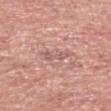{"biopsy_status": "not biopsied; imaged during a skin examination", "lesion_size": {"long_diameter_mm_approx": 3.5}, "lighting": "white-light", "patient": {"sex": "male", "age_approx": 65}, "image": {"source": "total-body photography crop", "field_of_view_mm": 15}, "site": "head or neck"}